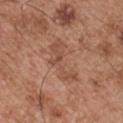Case summary:
• biopsy status: catalogued during a skin exam; not biopsied
• tile lighting: white-light illumination
• image-analysis metrics: a footprint of about 9 mm², an outline eccentricity of about 0.9 (0 = round, 1 = elongated), and a shape-asymmetry score of about 0.5 (0 = symmetric); an average lesion color of about L≈50 a*≈22 b*≈30 (CIELAB), roughly 7 lightness units darker than nearby skin, and a normalized border contrast of about 5
• location: the left upper arm
• patient: male, about 55 years old
• lesion diameter: about 5.5 mm
• acquisition: ~15 mm tile from a whole-body skin photo